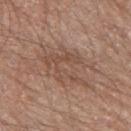Notes:
* biopsy status: imaged on a skin check; not biopsied
* image: ~15 mm crop, total-body skin-cancer survey
* TBP lesion metrics: an eccentricity of roughly 0.75 and two-axis asymmetry of about 0.35; border irregularity of about 6 on a 0–10 scale, internal color variation of about 4 on a 0–10 scale, and radial color variation of about 1.5
* body site: the left thigh
* subject: male, aged 58–62
* tile lighting: white-light illumination